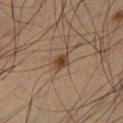biopsy_status: not biopsied; imaged during a skin examination
lighting: cross-polarized
image:
  source: total-body photography crop
  field_of_view_mm: 15
patient:
  sex: male
  age_approx: 65
lesion_size:
  long_diameter_mm_approx: 2.5
site: leg